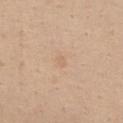notes = no biopsy performed (imaged during a skin exam) | lesion size = ≈1.5 mm | acquisition = ~15 mm tile from a whole-body skin photo | TBP lesion metrics = a footprint of about 1.5 mm², a shape eccentricity near 0.75, and two-axis asymmetry of about 0.25; a lesion color around L≈65 a*≈17 b*≈32 in CIELAB and a normalized lesion–skin contrast near 3.5; a border-irregularity rating of about 2/10, internal color variation of about 0 on a 0–10 scale, and radial color variation of about 0; an automated nevus-likeness rating near 0 out of 100 and a detector confidence of about 100 out of 100 that the crop contains a lesion | anatomic site = the front of the torso | subject = female, in their mid- to late 50s.